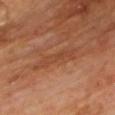workup: imaged on a skin check; not biopsied
image-analysis metrics: an outline eccentricity of about 0.95 (0 = round, 1 = elongated) and a shape-asymmetry score of about 0.35 (0 = symmetric); a classifier nevus-likeness of about 0/100
lesion diameter: about 5.5 mm
tile lighting: cross-polarized
body site: the upper back
patient: male, about 60 years old
image: ~15 mm crop, total-body skin-cancer survey